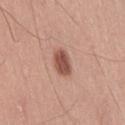The lesion was tiled from a total-body skin photograph and was not biopsied. The lesion is on the right thigh. Cropped from a whole-body photographic skin survey; the tile spans about 15 mm. Captured under white-light illumination. About 3.5 mm across. Automated image analysis of the tile measured roughly 14 lightness units darker than nearby skin and a normalized border contrast of about 9.5. The analysis additionally found a border-irregularity rating of about 2/10, a color-variation rating of about 2.5/10, and a peripheral color-asymmetry measure near 1. It also reported an automated nevus-likeness rating near 100 out of 100 and a lesion-detection confidence of about 100/100. A male subject aged 73 to 77.